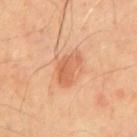patient: male, approximately 65 years of age; site: the front of the torso; acquisition: 15 mm crop, total-body photography.